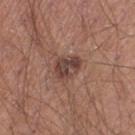Captured during whole-body skin photography for melanoma surveillance; the lesion was not biopsied. On the left thigh. This image is a 15 mm lesion crop taken from a total-body photograph. The patient is a male roughly 40 years of age.